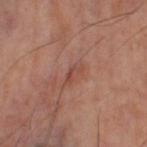No biopsy was performed on this lesion — it was imaged during a full skin examination and was not determined to be concerning. A close-up tile cropped from a whole-body skin photograph, about 15 mm across. From the right thigh. An algorithmic analysis of the crop reported a lesion area of about 2.5 mm² and a shape-asymmetry score of about 0.4 (0 = symmetric). The software also gave a lesion color around L≈47 a*≈24 b*≈27 in CIELAB, roughly 7 lightness units darker than nearby skin, and a normalized border contrast of about 5.5. The software also gave a classifier nevus-likeness of about 0/100 and a detector confidence of about 90 out of 100 that the crop contains a lesion. About 2.5 mm across. Imaged with cross-polarized lighting.